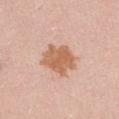follow-up — imaged on a skin check; not biopsied | imaging modality — 15 mm crop, total-body photography | subject — female, aged 13 to 17 | location — the abdomen | lesion diameter — about 4 mm.